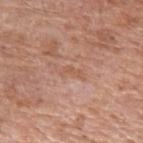Clinical impression: The lesion was tiled from a total-body skin photograph and was not biopsied. Image and clinical context: The lesion is on the left upper arm. Imaged with white-light lighting. The subject is a male aged 58 to 62. Approximately 2.5 mm at its widest. A lesion tile, about 15 mm wide, cut from a 3D total-body photograph.